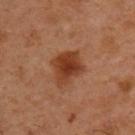Case summary:
* workup · total-body-photography surveillance lesion; no biopsy
* patient · male, in their mid- to late 50s
* tile lighting · cross-polarized illumination
* TBP lesion metrics · a lesion area of about 11 mm² and two-axis asymmetry of about 0.3; an average lesion color of about L≈38 a*≈25 b*≈33 (CIELAB), about 11 CIELAB-L* units darker than the surrounding skin, and a lesion-to-skin contrast of about 9.5 (normalized; higher = more distinct); a border-irregularity rating of about 3/10, internal color variation of about 3.5 on a 0–10 scale, and radial color variation of about 1
* image source · total-body-photography crop, ~15 mm field of view
* location · the upper back